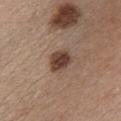Q: Was this lesion biopsied?
A: no biopsy performed (imaged during a skin exam)
Q: How large is the lesion?
A: ~3 mm (longest diameter)
Q: Where on the body is the lesion?
A: the chest
Q: What is the imaging modality?
A: 15 mm crop, total-body photography
Q: What lighting was used for the tile?
A: white-light illumination
Q: What are the patient's age and sex?
A: female, aged 43 to 47